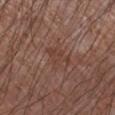{
  "biopsy_status": "not biopsied; imaged during a skin examination",
  "lighting": "white-light",
  "site": "left forearm",
  "patient": {
    "sex": "male",
    "age_approx": 75
  },
  "lesion_size": {
    "long_diameter_mm_approx": 4.0
  },
  "image": {
    "source": "total-body photography crop",
    "field_of_view_mm": 15
  }
}